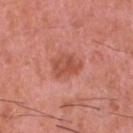The lesion's longest dimension is about 3.5 mm. A 15 mm crop from a total-body photograph taken for skin-cancer surveillance. The tile uses white-light illumination. The subject is a male aged around 45. The lesion-visualizer software estimated an average lesion color of about L≈52 a*≈30 b*≈32 (CIELAB), roughly 9 lightness units darker than nearby skin, and a normalized border contrast of about 7. The lesion is located on the head or neck.